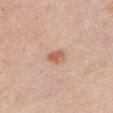Impression: Part of a total-body skin-imaging series; this lesion was reviewed on a skin check and was not flagged for biopsy. Image and clinical context: A female subject, in their 50s. A lesion tile, about 15 mm wide, cut from a 3D total-body photograph. Measured at roughly 2.5 mm in maximum diameter. Located on the chest. This is a white-light tile. An algorithmic analysis of the crop reported a lesion–skin lightness drop of about 10 and a normalized border contrast of about 7.5. The analysis additionally found a border-irregularity index near 2/10, a color-variation rating of about 3/10, and a peripheral color-asymmetry measure near 1.